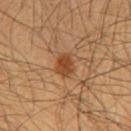Case summary:
* biopsy status: no biopsy performed (imaged during a skin exam)
* imaging modality: 15 mm crop, total-body photography
* body site: the chest
* patient: male, aged approximately 60
* lesion size: ~3 mm (longest diameter)
* lighting: cross-polarized illumination
* automated lesion analysis: a lesion color around L≈41 a*≈22 b*≈34 in CIELAB, a lesion–skin lightness drop of about 10, and a normalized lesion–skin contrast near 8; a border-irregularity rating of about 2.5/10, a color-variation rating of about 3.5/10, and radial color variation of about 1.5; a nevus-likeness score of about 95/100